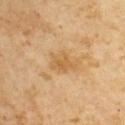The lesion was photographed on a routine skin check and not biopsied; there is no pathology result. Cropped from a total-body skin-imaging series; the visible field is about 15 mm. A male patient aged 63–67.